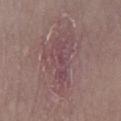workup: imaged on a skin check; not biopsied
image: 15 mm crop, total-body photography
location: the right lower leg
subject: male, in their mid-50s
tile lighting: white-light illumination